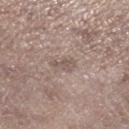Clinical impression:
This lesion was catalogued during total-body skin photography and was not selected for biopsy.
Acquisition and patient details:
From the leg. A male subject aged approximately 70. Automated image analysis of the tile measured a footprint of about 2.5 mm² and a shape eccentricity near 0.85. It also reported a nevus-likeness score of about 0/100 and a lesion-detection confidence of about 55/100. A roughly 15 mm field-of-view crop from a total-body skin photograph.